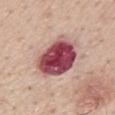Clinical impression: The lesion was tiled from a total-body skin photograph and was not biopsied. Clinical summary: The patient is a male roughly 55 years of age. Captured under white-light illumination. Longest diameter approximately 5.5 mm. On the mid back. An algorithmic analysis of the crop reported a lesion area of about 22 mm², an outline eccentricity of about 0.55 (0 = round, 1 = elongated), and a symmetry-axis asymmetry near 0.2. The software also gave a color-variation rating of about 9/10 and radial color variation of about 2.5. The analysis additionally found a nevus-likeness score of about 0/100. A roughly 15 mm field-of-view crop from a total-body skin photograph.